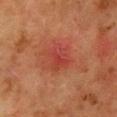follow-up: imaged on a skin check; not biopsied | illumination: cross-polarized | location: the chest | subject: female, aged 48–52 | size: ≈3.5 mm | image source: total-body-photography crop, ~15 mm field of view.